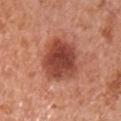notes — catalogued during a skin exam; not biopsied
patient — male, aged approximately 65
imaging modality — 15 mm crop, total-body photography
tile lighting — white-light
anatomic site — the chest
size — ≈5.5 mm
image-analysis metrics — two-axis asymmetry of about 0.2; a mean CIELAB color near L≈46 a*≈29 b*≈30, roughly 14 lightness units darker than nearby skin, and a lesion-to-skin contrast of about 10 (normalized; higher = more distinct); border irregularity of about 2 on a 0–10 scale and a color-variation rating of about 5/10